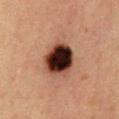The lesion is on the chest.
A female subject, roughly 40 years of age.
Cropped from a total-body skin-imaging series; the visible field is about 15 mm.
Approximately 4.5 mm at its widest.
The tile uses cross-polarized illumination.